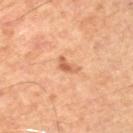  biopsy_status: not biopsied; imaged during a skin examination
  patient:
    sex: male
    age_approx: 50
  site: left leg
  image:
    source: total-body photography crop
    field_of_view_mm: 15